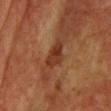Q: Illumination type?
A: cross-polarized illumination
Q: Who is the patient?
A: female, roughly 55 years of age
Q: How was this image acquired?
A: total-body-photography crop, ~15 mm field of view
Q: Where on the body is the lesion?
A: the chest
Q: Automated lesion metrics?
A: a mean CIELAB color near L≈29 a*≈22 b*≈27, roughly 8 lightness units darker than nearby skin, and a lesion-to-skin contrast of about 8.5 (normalized; higher = more distinct)